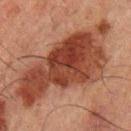location — the arm
image-analysis metrics — a footprint of about 55 mm², a shape eccentricity near 0.9, and two-axis asymmetry of about 0.4; a mean CIELAB color near L≈33 a*≈21 b*≈25, a lesion–skin lightness drop of about 12, and a normalized border contrast of about 10.5; border irregularity of about 6 on a 0–10 scale, internal color variation of about 5.5 on a 0–10 scale, and radial color variation of about 2; a nevus-likeness score of about 15/100 and lesion-presence confidence of about 100/100
tile lighting — cross-polarized
image source — total-body-photography crop, ~15 mm field of view
patient — male, aged around 50